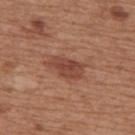follow-up: imaged on a skin check; not biopsied | size: ≈4.5 mm | patient: male, aged around 65 | illumination: white-light illumination | image: 15 mm crop, total-body photography | site: the upper back | automated metrics: an area of roughly 7.5 mm², a shape eccentricity near 0.85, and a shape-asymmetry score of about 0.25 (0 = symmetric); a border-irregularity rating of about 3/10 and a within-lesion color-variation index near 2.5/10; a classifier nevus-likeness of about 80/100 and a detector confidence of about 100 out of 100 that the crop contains a lesion.